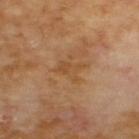Assessment:
Imaged during a routine full-body skin examination; the lesion was not biopsied and no histopathology is available.
Acquisition and patient details:
Automated tile analysis of the lesion measured a mean CIELAB color near L≈48 a*≈20 b*≈37 and a normalized border contrast of about 5.5. It also reported lesion-presence confidence of about 100/100. A close-up tile cropped from a whole-body skin photograph, about 15 mm across. A male subject aged approximately 70. The tile uses cross-polarized illumination. The lesion's longest dimension is about 3.5 mm. The lesion is located on the upper back.